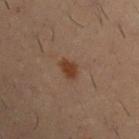This lesion was catalogued during total-body skin photography and was not selected for biopsy.
The tile uses cross-polarized illumination.
The lesion is located on the arm.
Cropped from a whole-body photographic skin survey; the tile spans about 15 mm.
The lesion-visualizer software estimated a lesion color around L≈30 a*≈16 b*≈25 in CIELAB, about 8 CIELAB-L* units darker than the surrounding skin, and a normalized border contrast of about 9.
Approximately 2.5 mm at its widest.
A male patient, aged approximately 30.